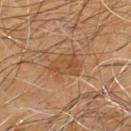Imaged during a routine full-body skin examination; the lesion was not biopsied and no histopathology is available. Measured at roughly 4 mm in maximum diameter. This is a cross-polarized tile. The subject is a male roughly 60 years of age. Located on the chest. A 15 mm close-up tile from a total-body photography series done for melanoma screening.